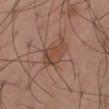Image and clinical context: Captured under cross-polarized illumination. The lesion is located on the right thigh. A male subject, approximately 55 years of age. Cropped from a total-body skin-imaging series; the visible field is about 15 mm. Approximately 4 mm at its widest.